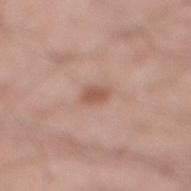<record>
<automated_metrics>
  <cielab_L>55</cielab_L>
  <cielab_a>21</cielab_a>
  <cielab_b>27</cielab_b>
  <vs_skin_darker_L>10.0</vs_skin_darker_L>
  <vs_skin_contrast_norm>7.0</vs_skin_contrast_norm>
  <border_irregularity_0_10>2.5</border_irregularity_0_10>
  <color_variation_0_10>1.5</color_variation_0_10>
  <peripheral_color_asymmetry>0.5</peripheral_color_asymmetry>
</automated_metrics>
<image>
  <source>total-body photography crop</source>
  <field_of_view_mm>15</field_of_view_mm>
</image>
<lighting>white-light</lighting>
<lesion_size>
  <long_diameter_mm_approx>2.5</long_diameter_mm_approx>
</lesion_size>
<site>right lower leg</site>
<patient>
  <sex>male</sex>
  <age_approx>40</age_approx>
</patient>
</record>